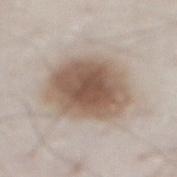{
  "biopsy_status": "not biopsied; imaged during a skin examination",
  "lighting": "white-light",
  "site": "front of the torso",
  "image": {
    "source": "total-body photography crop",
    "field_of_view_mm": 15
  },
  "patient": {
    "sex": "male",
    "age_approx": 80
  }
}